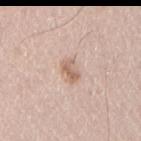notes: no biopsy performed (imaged during a skin exam)
image-analysis metrics: a mean CIELAB color near L≈63 a*≈17 b*≈28 and a normalized border contrast of about 7; an automated nevus-likeness rating near 30 out of 100 and a lesion-detection confidence of about 100/100
lesion size: ≈2.5 mm
patient: male, aged 43–47
illumination: white-light illumination
imaging modality: ~15 mm crop, total-body skin-cancer survey
site: the right upper arm